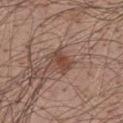{
  "biopsy_status": "not biopsied; imaged during a skin examination",
  "image": {
    "source": "total-body photography crop",
    "field_of_view_mm": 15
  },
  "automated_metrics": {
    "border_irregularity_0_10": 2.0,
    "color_variation_0_10": 2.0,
    "peripheral_color_asymmetry": 0.5
  },
  "lighting": "white-light",
  "site": "mid back",
  "patient": {
    "sex": "male",
    "age_approx": 50
  },
  "lesion_size": {
    "long_diameter_mm_approx": 3.0
  }
}